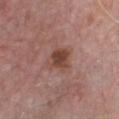Assessment: The lesion was tiled from a total-body skin photograph and was not biopsied. Context: Approximately 3 mm at its widest. A male patient, about 65 years old. The lesion is on the chest. Automated tile analysis of the lesion measured a lesion area of about 6.5 mm², an eccentricity of roughly 0.4, and a symmetry-axis asymmetry near 0.3. The software also gave internal color variation of about 4 on a 0–10 scale and a peripheral color-asymmetry measure near 1.5. It also reported a nevus-likeness score of about 50/100. This is a white-light tile. A close-up tile cropped from a whole-body skin photograph, about 15 mm across.